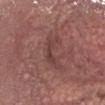This lesion was catalogued during total-body skin photography and was not selected for biopsy. This is a white-light tile. The lesion is located on the chest. A 15 mm close-up tile from a total-body photography series done for melanoma screening. A female subject aged 33–37. About 4 mm across.